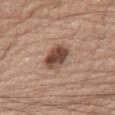{
  "biopsy_status": "not biopsied; imaged during a skin examination",
  "lesion_size": {
    "long_diameter_mm_approx": 4.0
  },
  "patient": {
    "sex": "male",
    "age_approx": 75
  },
  "site": "front of the torso",
  "lighting": "white-light",
  "image": {
    "source": "total-body photography crop",
    "field_of_view_mm": 15
  }
}